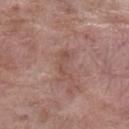Notes:
• biopsy status · no biopsy performed (imaged during a skin exam)
• subject · female, in their mid-80s or older
• anatomic site · the left lower leg
• imaging modality · total-body-photography crop, ~15 mm field of view
• illumination · white-light
• TBP lesion metrics · a mean CIELAB color near L≈50 a*≈21 b*≈25, about 7 CIELAB-L* units darker than the surrounding skin, and a normalized border contrast of about 5; a within-lesion color-variation index near 0/10 and radial color variation of about 0; an automated nevus-likeness rating near 0 out of 100 and a detector confidence of about 100 out of 100 that the crop contains a lesion
• diameter · ≈3.5 mm